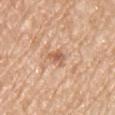{"biopsy_status": "not biopsied; imaged during a skin examination", "lighting": "white-light", "image": {"source": "total-body photography crop", "field_of_view_mm": 15}, "patient": {"sex": "male", "age_approx": 60}, "site": "chest"}